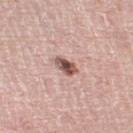Impression: The lesion was photographed on a routine skin check and not biopsied; there is no pathology result. Clinical summary: Approximately 3 mm at its widest. The patient is a male about 75 years old. The lesion is on the leg. A lesion tile, about 15 mm wide, cut from a 3D total-body photograph. Captured under white-light illumination. The lesion-visualizer software estimated an area of roughly 4 mm², a shape eccentricity near 0.85, and two-axis asymmetry of about 0.2. It also reported a border-irregularity index near 2/10, internal color variation of about 5 on a 0–10 scale, and peripheral color asymmetry of about 1. The software also gave a lesion-detection confidence of about 100/100.